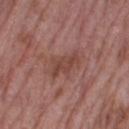biopsy_status: not biopsied; imaged during a skin examination
patient:
  sex: female
  age_approx: 70
image:
  source: total-body photography crop
  field_of_view_mm: 15
lesion_size:
  long_diameter_mm_approx: 4.0
site: leg
lighting: white-light